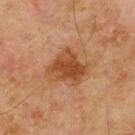Imaged during a routine full-body skin examination; the lesion was not biopsied and no histopathology is available.
Captured under cross-polarized illumination.
A male subject, aged 68 to 72.
Cropped from a whole-body photographic skin survey; the tile spans about 15 mm.
An algorithmic analysis of the crop reported an average lesion color of about L≈39 a*≈20 b*≈32 (CIELAB) and about 9 CIELAB-L* units darker than the surrounding skin. The software also gave a nevus-likeness score of about 40/100 and lesion-presence confidence of about 100/100.
Approximately 4 mm at its widest.
On the upper back.